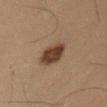  lighting: cross-polarized
  patient:
    sex: male
    age_approx: 55
  site: arm
  image:
    source: total-body photography crop
    field_of_view_mm: 15
  automated_metrics:
    vs_skin_darker_L: 11.0
    vs_skin_contrast_norm: 10.5
    border_irregularity_0_10: 2.0
    color_variation_0_10: 3.0